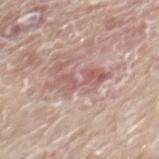notes: no biopsy performed (imaged during a skin exam)
anatomic site: the mid back
illumination: white-light illumination
automated metrics: a lesion area of about 8 mm² and a shape-asymmetry score of about 0.4 (0 = symmetric); roughly 10 lightness units darker than nearby skin and a lesion-to-skin contrast of about 6.5 (normalized; higher = more distinct)
diameter: ≈6 mm
image source: ~15 mm tile from a whole-body skin photo
patient: male, in their mid- to late 60s